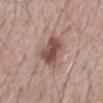* workup · total-body-photography surveillance lesion; no biopsy
* illumination · white-light illumination
* anatomic site · the abdomen
* TBP lesion metrics · a shape-asymmetry score of about 0.3 (0 = symmetric); a border-irregularity rating of about 3/10, a color-variation rating of about 4.5/10, and peripheral color asymmetry of about 1.5; an automated nevus-likeness rating near 75 out of 100 and lesion-presence confidence of about 100/100
* lesion size · ~4.5 mm (longest diameter)
* patient · male, aged 68 to 72
* imaging modality · ~15 mm tile from a whole-body skin photo